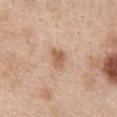biopsy_status: not biopsied; imaged during a skin examination
automated_metrics:
  area_mm2_approx: 4.5
  shape_asymmetry: 0.35
  cielab_L: 60
  cielab_a: 21
  cielab_b: 33
  vs_skin_darker_L: 11.0
  vs_skin_contrast_norm: 7.5
  border_irregularity_0_10: 3.0
  color_variation_0_10: 2.5
  peripheral_color_asymmetry: 1.0
  nevus_likeness_0_100: 80
  lesion_detection_confidence_0_100: 100
image:
  source: total-body photography crop
  field_of_view_mm: 15
patient:
  sex: female
  age_approx: 40
site: abdomen
lighting: white-light
lesion_size:
  long_diameter_mm_approx: 3.0